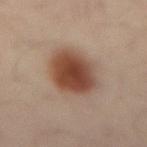Q: What is the lesion's diameter?
A: ~5.5 mm (longest diameter)
Q: Patient demographics?
A: male, about 40 years old
Q: How was the tile lit?
A: cross-polarized illumination
Q: What is the imaging modality?
A: total-body-photography crop, ~15 mm field of view
Q: Lesion location?
A: the back
Q: Automated lesion metrics?
A: a shape eccentricity near 0.65 and a shape-asymmetry score of about 0.15 (0 = symmetric); border irregularity of about 1.5 on a 0–10 scale and a peripheral color-asymmetry measure near 1; a classifier nevus-likeness of about 100/100 and lesion-presence confidence of about 100/100